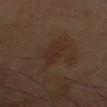Clinical impression:
Captured during whole-body skin photography for melanoma surveillance; the lesion was not biopsied.
Context:
A 15 mm close-up extracted from a 3D total-body photography capture. This is a cross-polarized tile. The lesion's longest dimension is about 4 mm. The total-body-photography lesion software estimated an area of roughly 6.5 mm², a shape eccentricity near 0.85, and a shape-asymmetry score of about 0.35 (0 = symmetric). The analysis additionally found an automated nevus-likeness rating near 0 out of 100 and lesion-presence confidence of about 100/100. The lesion is on the right forearm. The patient is a male roughly 70 years of age.